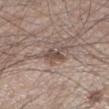Case summary:
* workup — imaged on a skin check; not biopsied